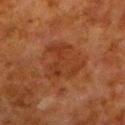Part of a total-body skin-imaging series; this lesion was reviewed on a skin check and was not flagged for biopsy.
A 15 mm close-up extracted from a 3D total-body photography capture.
The lesion is located on the leg.
About 6 mm across.
The lesion-visualizer software estimated an area of roughly 19 mm², a shape eccentricity near 0.6, and a shape-asymmetry score of about 0.35 (0 = symmetric). It also reported a mean CIELAB color near L≈30 a*≈21 b*≈29, a lesion–skin lightness drop of about 5, and a lesion-to-skin contrast of about 5.5 (normalized; higher = more distinct). The analysis additionally found border irregularity of about 4.5 on a 0–10 scale, a within-lesion color-variation index near 3/10, and a peripheral color-asymmetry measure near 1. The software also gave an automated nevus-likeness rating near 0 out of 100 and a lesion-detection confidence of about 100/100.
A male patient aged 78 to 82.